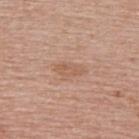Captured during whole-body skin photography for melanoma surveillance; the lesion was not biopsied.